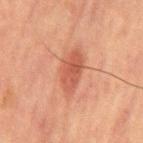A 15 mm crop from a total-body photograph taken for skin-cancer surveillance. A male patient, aged 63–67. The recorded lesion diameter is about 5 mm. The tile uses cross-polarized illumination. Located on the abdomen.